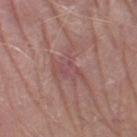Assessment:
The lesion was tiled from a total-body skin photograph and was not biopsied.
Background:
The lesion is located on the left lower leg. A male subject, about 65 years old. Cropped from a total-body skin-imaging series; the visible field is about 15 mm. The total-body-photography lesion software estimated lesion-presence confidence of about 70/100.